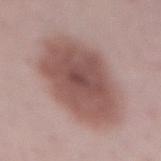Clinical impression: The lesion was tiled from a total-body skin photograph and was not biopsied. Acquisition and patient details: Imaged with white-light lighting. A lesion tile, about 15 mm wide, cut from a 3D total-body photograph. A female subject aged around 50. Located on the abdomen. The lesion's longest dimension is about 8 mm. The lesion-visualizer software estimated a footprint of about 43 mm² and a symmetry-axis asymmetry near 0.15. It also reported an average lesion color of about L≈52 a*≈20 b*≈22 (CIELAB), about 13 CIELAB-L* units darker than the surrounding skin, and a normalized lesion–skin contrast near 9. And it measured border irregularity of about 1.5 on a 0–10 scale, a within-lesion color-variation index near 4/10, and peripheral color asymmetry of about 1. It also reported a classifier nevus-likeness of about 65/100 and lesion-presence confidence of about 100/100.